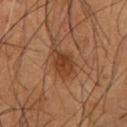location: the right forearm | illumination: cross-polarized | image source: ~15 mm tile from a whole-body skin photo | lesion size: ≈4.5 mm | subject: male, roughly 55 years of age.